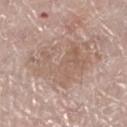The lesion was photographed on a routine skin check and not biopsied; there is no pathology result.
The total-body-photography lesion software estimated a lesion color around L≈58 a*≈16 b*≈26 in CIELAB and roughly 7 lightness units darker than nearby skin. The analysis additionally found a classifier nevus-likeness of about 0/100 and a lesion-detection confidence of about 90/100.
On the right leg.
A male patient roughly 70 years of age.
About 6.5 mm across.
This is a white-light tile.
A 15 mm close-up extracted from a 3D total-body photography capture.